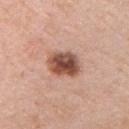Captured during whole-body skin photography for melanoma surveillance; the lesion was not biopsied. A close-up tile cropped from a whole-body skin photograph, about 15 mm across. A male subject, roughly 60 years of age. The total-body-photography lesion software estimated a footprint of about 10 mm², an eccentricity of roughly 0.65, and two-axis asymmetry of about 0.15. Longest diameter approximately 4 mm. On the front of the torso. Imaged with white-light lighting.